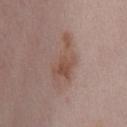– follow-up — total-body-photography surveillance lesion; no biopsy
– tile lighting — white-light
– acquisition — ~15 mm tile from a whole-body skin photo
– anatomic site — the front of the torso
– diameter — ~6.5 mm (longest diameter)
– patient — female, in their 50s
– automated metrics — a border-irregularity index near 5/10, a within-lesion color-variation index near 4.5/10, and peripheral color asymmetry of about 1; a classifier nevus-likeness of about 15/100